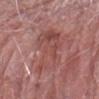The lesion was tiled from a total-body skin photograph and was not biopsied. On the right forearm. The subject is a male about 75 years old. This is a white-light tile. A roughly 15 mm field-of-view crop from a total-body skin photograph. Automated tile analysis of the lesion measured border irregularity of about 6 on a 0–10 scale, internal color variation of about 6 on a 0–10 scale, and a peripheral color-asymmetry measure near 2.5. About 8 mm across.